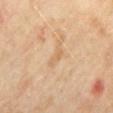biopsy_status: not biopsied; imaged during a skin examination
patient:
  sex: male
  age_approx: 65
image:
  source: total-body photography crop
  field_of_view_mm: 15
site: mid back